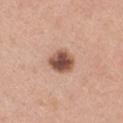The lesion is located on the left upper arm. The lesion's longest dimension is about 3 mm. This is a white-light tile. A 15 mm close-up tile from a total-body photography series done for melanoma screening. A male patient, aged 58 to 62.